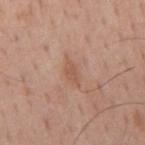Part of a total-body skin-imaging series; this lesion was reviewed on a skin check and was not flagged for biopsy.
The lesion is on the back.
The total-body-photography lesion software estimated a lesion color around L≈54 a*≈22 b*≈29 in CIELAB, a lesion–skin lightness drop of about 7, and a normalized border contrast of about 5.5.
Approximately 2.5 mm at its widest.
A male subject aged around 60.
A 15 mm crop from a total-body photograph taken for skin-cancer surveillance.
This is a white-light tile.